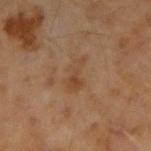workup = total-body-photography surveillance lesion; no biopsy | image source = ~15 mm crop, total-body skin-cancer survey | tile lighting = cross-polarized | body site = the arm | patient = male, in their mid- to late 40s | lesion diameter = about 4 mm.